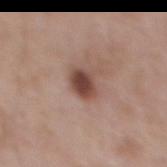Clinical summary: The subject is a male aged 53–57. The lesion is located on the mid back. A region of skin cropped from a whole-body photographic capture, roughly 15 mm wide.